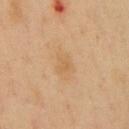Assessment:
Imaged during a routine full-body skin examination; the lesion was not biopsied and no histopathology is available.
Context:
Measured at roughly 2.5 mm in maximum diameter. The patient is a male aged around 50. This is a cross-polarized tile. Automated image analysis of the tile measured a lesion area of about 3.5 mm², an eccentricity of roughly 0.75, and two-axis asymmetry of about 0.35. The software also gave a border-irregularity rating of about 3.5/10, a color-variation rating of about 1.5/10, and peripheral color asymmetry of about 0.5. It also reported a lesion-detection confidence of about 100/100. The lesion is located on the chest. This image is a 15 mm lesion crop taken from a total-body photograph.